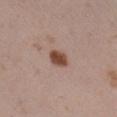Recorded during total-body skin imaging; not selected for excision or biopsy.
Located on the right lower leg.
Imaged with white-light lighting.
The lesion's longest dimension is about 3 mm.
A female subject about 30 years old.
A roughly 15 mm field-of-view crop from a total-body skin photograph.